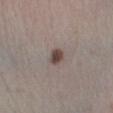| feature | finding |
|---|---|
| biopsy status | total-body-photography surveillance lesion; no biopsy |
| patient | female, roughly 55 years of age |
| TBP lesion metrics | a border-irregularity index near 1/10 and a within-lesion color-variation index near 2.5/10; a nevus-likeness score of about 95/100 and lesion-presence confidence of about 100/100 |
| body site | the left lower leg |
| acquisition | 15 mm crop, total-body photography |